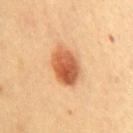{"biopsy_status": "not biopsied; imaged during a skin examination", "site": "chest", "patient": {"sex": "female", "age_approx": 65}, "lesion_size": {"long_diameter_mm_approx": 5.0}, "lighting": "cross-polarized", "image": {"source": "total-body photography crop", "field_of_view_mm": 15}}